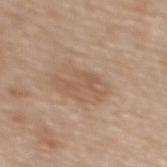Captured during whole-body skin photography for melanoma surveillance; the lesion was not biopsied. From the upper back. Longest diameter approximately 3.5 mm. A 15 mm close-up tile from a total-body photography series done for melanoma screening. A female subject, approximately 65 years of age. Automated tile analysis of the lesion measured border irregularity of about 9.5 on a 0–10 scale and internal color variation of about 0 on a 0–10 scale.